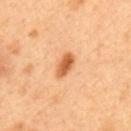Recorded during total-body skin imaging; not selected for excision or biopsy.
A male subject, aged 48 to 52.
The recorded lesion diameter is about 3.5 mm.
Automated tile analysis of the lesion measured a mean CIELAB color near L≈62 a*≈27 b*≈43 and about 14 CIELAB-L* units darker than the surrounding skin. It also reported a border-irregularity rating of about 2/10, a color-variation rating of about 3.5/10, and peripheral color asymmetry of about 1.5. And it measured an automated nevus-likeness rating near 95 out of 100 and a detector confidence of about 100 out of 100 that the crop contains a lesion.
Cropped from a whole-body photographic skin survey; the tile spans about 15 mm.
From the upper back.
This is a cross-polarized tile.